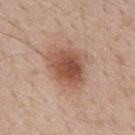Assessment:
The lesion was photographed on a routine skin check and not biopsied; there is no pathology result.
Clinical summary:
The lesion-visualizer software estimated a footprint of about 15 mm² and a shape-asymmetry score of about 0.25 (0 = symmetric). And it measured a lesion color around L≈52 a*≈22 b*≈29 in CIELAB, roughly 13 lightness units darker than nearby skin, and a lesion-to-skin contrast of about 9 (normalized; higher = more distinct). The recorded lesion diameter is about 5 mm. Cropped from a whole-body photographic skin survey; the tile spans about 15 mm. A male subject, aged around 50. The tile uses white-light illumination. On the mid back.